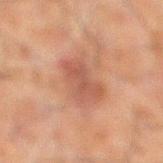follow-up — imaged on a skin check; not biopsied | subject — male, in their 60s | image source — total-body-photography crop, ~15 mm field of view | lesion diameter — ~4 mm (longest diameter) | tile lighting — cross-polarized | site — the left leg | TBP lesion metrics — a shape eccentricity near 0.65; a border-irregularity rating of about 3/10 and radial color variation of about 0.5.